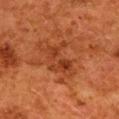| key | value |
|---|---|
| follow-up | catalogued during a skin exam; not biopsied |
| location | the upper back |
| subject | female, aged approximately 50 |
| imaging modality | ~15 mm crop, total-body skin-cancer survey |